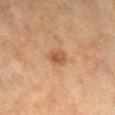Part of a total-body skin-imaging series; this lesion was reviewed on a skin check and was not flagged for biopsy. The lesion is located on the right lower leg. The lesion-visualizer software estimated a footprint of about 4 mm², an eccentricity of roughly 0.7, and two-axis asymmetry of about 0.25. It also reported an average lesion color of about L≈51 a*≈22 b*≈34 (CIELAB). It also reported a border-irregularity rating of about 2/10, internal color variation of about 3 on a 0–10 scale, and radial color variation of about 1. The software also gave a lesion-detection confidence of about 100/100. A female patient approximately 60 years of age. Approximately 2.5 mm at its widest. A 15 mm close-up tile from a total-body photography series done for melanoma screening.